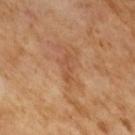Clinical impression: The lesion was photographed on a routine skin check and not biopsied; there is no pathology result. Acquisition and patient details: This is a cross-polarized tile. A 15 mm close-up tile from a total-body photography series done for melanoma screening. A male patient, aged around 65. The recorded lesion diameter is about 3.5 mm.